From the mid back, a roughly 15 mm field-of-view crop from a total-body skin photograph, the patient is a male aged around 65 — 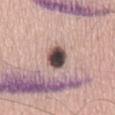Q: How was the tile lit?
A: white-light
Q: What did pathology find?
A: a dysplastic (Clark) nevus — a benign lesion